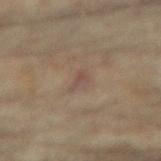Captured during whole-body skin photography for melanoma surveillance; the lesion was not biopsied.
A female patient approximately 80 years of age.
The total-body-photography lesion software estimated a footprint of about 3 mm² and a shape-asymmetry score of about 0.5 (0 = symmetric). It also reported a lesion color around L≈43 a*≈14 b*≈21 in CIELAB and a lesion–skin lightness drop of about 6. It also reported a border-irregularity index near 4.5/10 and a peripheral color-asymmetry measure near 0.5.
From the right arm.
Captured under cross-polarized illumination.
This image is a 15 mm lesion crop taken from a total-body photograph.
The lesion's longest dimension is about 2.5 mm.